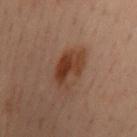Notes:
– follow-up · total-body-photography surveillance lesion; no biopsy